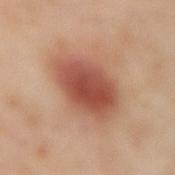workup = imaged on a skin check; not biopsied
patient = female, about 55 years old
image source = ~15 mm tile from a whole-body skin photo
lighting = cross-polarized
lesion diameter = ~6.5 mm (longest diameter)
body site = the back